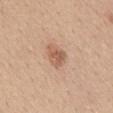Case summary:
- follow-up · no biopsy performed (imaged during a skin exam)
- illumination · white-light
- subject · female, in their mid- to late 40s
- lesion size · ≈3.5 mm
- TBP lesion metrics · a shape eccentricity near 0.8; an average lesion color of about L≈59 a*≈21 b*≈31 (CIELAB), roughly 10 lightness units darker than nearby skin, and a lesion-to-skin contrast of about 7 (normalized; higher = more distinct); an automated nevus-likeness rating near 75 out of 100 and a lesion-detection confidence of about 100/100
- site · the mid back
- acquisition · 15 mm crop, total-body photography